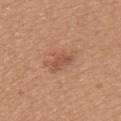notes: catalogued during a skin exam; not biopsied | diameter: ~5 mm (longest diameter) | site: the upper back | image source: ~15 mm tile from a whole-body skin photo | image-analysis metrics: a mean CIELAB color near L≈54 a*≈22 b*≈32, a lesion–skin lightness drop of about 8, and a lesion-to-skin contrast of about 5.5 (normalized; higher = more distinct); an automated nevus-likeness rating near 30 out of 100 and a detector confidence of about 100 out of 100 that the crop contains a lesion | illumination: white-light | patient: female, roughly 30 years of age.